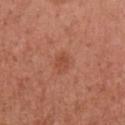Findings:
- workup: no biopsy performed (imaged during a skin exam)
- patient: female, aged 48–52
- location: the arm
- imaging modality: total-body-photography crop, ~15 mm field of view